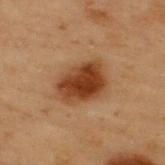<tbp_lesion>
  <biopsy_status>not biopsied; imaged during a skin examination</biopsy_status>
  <lesion_size>
    <long_diameter_mm_approx>5.0</long_diameter_mm_approx>
  </lesion_size>
  <image>
    <source>total-body photography crop</source>
    <field_of_view_mm>15</field_of_view_mm>
  </image>
  <site>upper back</site>
  <patient>
    <sex>male</sex>
    <age_approx>55</age_approx>
  </patient>
</tbp_lesion>